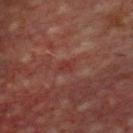| key | value |
|---|---|
| size | about 2.5 mm |
| image source | ~15 mm crop, total-body skin-cancer survey |
| subject | male, in their mid- to late 60s |
| tile lighting | cross-polarized illumination |
| site | the upper back |
| automated lesion analysis | a lesion area of about 3 mm², an outline eccentricity of about 0.85 (0 = round, 1 = elongated), and a symmetry-axis asymmetry near 0.3; border irregularity of about 2.5 on a 0–10 scale, a color-variation rating of about 1.5/10, and radial color variation of about 0.5; lesion-presence confidence of about 80/100 |